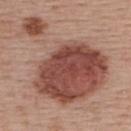image source: 15 mm crop, total-body photography | anatomic site: the upper back | subject: female, aged 53–57 | lighting: white-light illumination | size: about 12 mm.